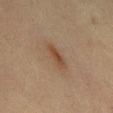Impression: Captured during whole-body skin photography for melanoma surveillance; the lesion was not biopsied.